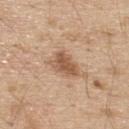Case summary:
• biopsy status · total-body-photography surveillance lesion; no biopsy
• body site · the upper back
• lesion diameter · about 3.5 mm
• lighting · white-light
• patient · male, aged around 70
• imaging modality · 15 mm crop, total-body photography
• automated lesion analysis · a lesion area of about 8 mm², a shape eccentricity near 0.7, and a shape-asymmetry score of about 0.25 (0 = symmetric); border irregularity of about 3 on a 0–10 scale, internal color variation of about 3 on a 0–10 scale, and radial color variation of about 1